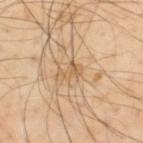Clinical impression: Part of a total-body skin-imaging series; this lesion was reviewed on a skin check and was not flagged for biopsy. Background: The total-body-photography lesion software estimated a within-lesion color-variation index near 1/10 and radial color variation of about 0.5. It also reported a lesion-detection confidence of about 85/100. From the back. This image is a 15 mm lesion crop taken from a total-body photograph. Captured under cross-polarized illumination. A male subject, aged around 55.